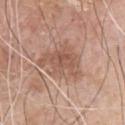Clinical summary: A male patient about 80 years old. A region of skin cropped from a whole-body photographic capture, roughly 15 mm wide. The lesion is located on the chest.